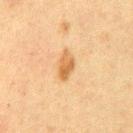Q: Is there a histopathology result?
A: no biopsy performed (imaged during a skin exam)
Q: How large is the lesion?
A: ~3.5 mm (longest diameter)
Q: What kind of image is this?
A: 15 mm crop, total-body photography
Q: What did automated image analysis measure?
A: an area of roughly 5 mm², an outline eccentricity of about 0.85 (0 = round, 1 = elongated), and a shape-asymmetry score of about 0.2 (0 = symmetric); a border-irregularity index near 2/10, a within-lesion color-variation index near 3.5/10, and a peripheral color-asymmetry measure near 1.5; a nevus-likeness score of about 75/100 and a lesion-detection confidence of about 100/100
Q: Illumination type?
A: cross-polarized illumination
Q: Patient demographics?
A: male, about 50 years old
Q: Where on the body is the lesion?
A: the front of the torso